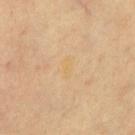Q: Is there a histopathology result?
A: total-body-photography surveillance lesion; no biopsy
Q: What are the patient's age and sex?
A: aged around 60
Q: Lesion location?
A: the chest
Q: How large is the lesion?
A: about 2.5 mm
Q: What kind of image is this?
A: 15 mm crop, total-body photography
Q: What lighting was used for the tile?
A: cross-polarized illumination